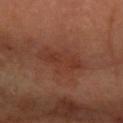No biopsy was performed on this lesion — it was imaged during a full skin examination and was not determined to be concerning.
On the right forearm.
A female subject.
Cropped from a total-body skin-imaging series; the visible field is about 15 mm.